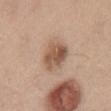The lesion was tiled from a total-body skin photograph and was not biopsied. Approximately 4 mm at its widest. The tile uses white-light illumination. A female subject, aged around 40. On the back. A roughly 15 mm field-of-view crop from a total-body skin photograph. The lesion-visualizer software estimated an area of roughly 10 mm² and an outline eccentricity of about 0.6 (0 = round, 1 = elongated). And it measured a mean CIELAB color near L≈54 a*≈18 b*≈29 and a normalized lesion–skin contrast near 8. The analysis additionally found internal color variation of about 5.5 on a 0–10 scale and a peripheral color-asymmetry measure near 2. And it measured a nevus-likeness score of about 35/100 and a lesion-detection confidence of about 100/100.